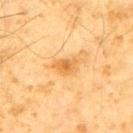No biopsy was performed on this lesion — it was imaged during a full skin examination and was not determined to be concerning. Automated tile analysis of the lesion measured an area of roughly 5.5 mm², an eccentricity of roughly 0.75, and a symmetry-axis asymmetry near 0.3. The analysis additionally found an average lesion color of about L≈56 a*≈21 b*≈43 (CIELAB) and a normalized lesion–skin contrast near 7. It also reported border irregularity of about 3 on a 0–10 scale and a within-lesion color-variation index near 3.5/10. The analysis additionally found an automated nevus-likeness rating near 20 out of 100 and lesion-presence confidence of about 100/100. From the left upper arm. A 15 mm crop from a total-body photograph taken for skin-cancer surveillance. Captured under cross-polarized illumination. A male patient aged 68 to 72.